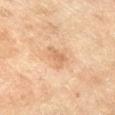Recorded during total-body skin imaging; not selected for excision or biopsy. A region of skin cropped from a whole-body photographic capture, roughly 15 mm wide. A female patient, aged 68–72. The tile uses cross-polarized illumination. The lesion-visualizer software estimated a shape eccentricity near 0.7 and a shape-asymmetry score of about 0.5 (0 = symmetric). The software also gave a nevus-likeness score of about 0/100 and lesion-presence confidence of about 100/100. The lesion is located on the right lower leg. Approximately 3 mm at its widest.